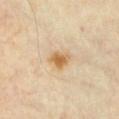No biopsy was performed on this lesion — it was imaged during a full skin examination and was not determined to be concerning. On the chest. Longest diameter approximately 3 mm. A male patient, approximately 65 years of age. A 15 mm close-up tile from a total-body photography series done for melanoma screening. Imaged with cross-polarized lighting.